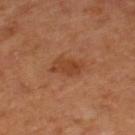Assessment:
Recorded during total-body skin imaging; not selected for excision or biopsy.
Image and clinical context:
A female subject aged 63–67. On the right lower leg. About 3.5 mm across. A 15 mm close-up extracted from a 3D total-body photography capture. The tile uses cross-polarized illumination.